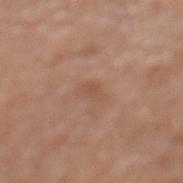Recorded during total-body skin imaging; not selected for excision or biopsy.
A lesion tile, about 15 mm wide, cut from a 3D total-body photograph.
A female patient, in their 80s.
On the mid back.